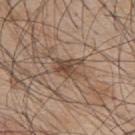Assessment: Imaged during a routine full-body skin examination; the lesion was not biopsied and no histopathology is available. Context: From the upper back. Measured at roughly 3.5 mm in maximum diameter. The subject is a male in their mid-40s. Automated image analysis of the tile measured a footprint of about 6 mm², a shape eccentricity near 0.7, and two-axis asymmetry of about 0.3. The analysis additionally found a lesion color around L≈47 a*≈16 b*≈27 in CIELAB, about 10 CIELAB-L* units darker than the surrounding skin, and a lesion-to-skin contrast of about 7.5 (normalized; higher = more distinct). The software also gave border irregularity of about 3 on a 0–10 scale and a color-variation rating of about 4/10. Cropped from a whole-body photographic skin survey; the tile spans about 15 mm. Imaged with white-light lighting.